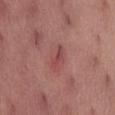Notes:
– biopsy status: imaged on a skin check; not biopsied
– image source: 15 mm crop, total-body photography
– diameter: ≈3 mm
– location: the abdomen
– patient: male, aged 68–72